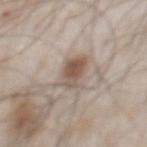<tbp_lesion>
<biopsy_status>not biopsied; imaged during a skin examination</biopsy_status>
</tbp_lesion>